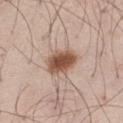No biopsy was performed on this lesion — it was imaged during a full skin examination and was not determined to be concerning.
The lesion's longest dimension is about 4 mm.
A male patient, aged 43–47.
The total-body-photography lesion software estimated a lesion area of about 9.5 mm², an eccentricity of roughly 0.55, and a symmetry-axis asymmetry near 0.2. It also reported a mean CIELAB color near L≈53 a*≈19 b*≈29 and a lesion-to-skin contrast of about 11 (normalized; higher = more distinct). The analysis additionally found a border-irregularity index near 2/10, a color-variation rating of about 4/10, and a peripheral color-asymmetry measure near 1. It also reported a nevus-likeness score of about 100/100 and lesion-presence confidence of about 100/100.
From the right thigh.
This image is a 15 mm lesion crop taken from a total-body photograph.